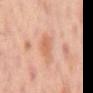Assessment: No biopsy was performed on this lesion — it was imaged during a full skin examination and was not determined to be concerning. Clinical summary: A region of skin cropped from a whole-body photographic capture, roughly 15 mm wide. Automated tile analysis of the lesion measured a mean CIELAB color near L≈59 a*≈23 b*≈32, roughly 7 lightness units darker than nearby skin, and a normalized lesion–skin contrast near 6. The analysis additionally found border irregularity of about 2.5 on a 0–10 scale, a color-variation rating of about 2.5/10, and a peripheral color-asymmetry measure near 1. The software also gave a lesion-detection confidence of about 100/100. This is a cross-polarized tile. The patient is a male aged approximately 60. The lesion's longest dimension is about 3 mm. From the back.